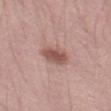Impression:
Part of a total-body skin-imaging series; this lesion was reviewed on a skin check and was not flagged for biopsy.
Acquisition and patient details:
About 3.5 mm across. A male subject approximately 30 years of age. A region of skin cropped from a whole-body photographic capture, roughly 15 mm wide. This is a white-light tile.